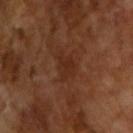Case summary:
* biopsy status — total-body-photography surveillance lesion; no biopsy
* patient — male, aged 63–67
* imaging modality — 15 mm crop, total-body photography
* lesion size — ≈3 mm